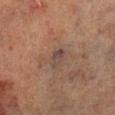Findings:
* biopsy status · catalogued during a skin exam; not biopsied
* site · the right lower leg
* illumination · cross-polarized
* subject · male, roughly 70 years of age
* image source · total-body-photography crop, ~15 mm field of view
* lesion size · ≈2.5 mm